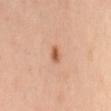Q: Lesion size?
A: ~2.5 mm (longest diameter)
Q: What is the imaging modality?
A: total-body-photography crop, ~15 mm field of view
Q: What is the anatomic site?
A: the mid back
Q: What lighting was used for the tile?
A: cross-polarized
Q: Patient demographics?
A: female, approximately 40 years of age